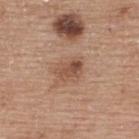follow-up: no biopsy performed (imaged during a skin exam) | body site: the upper back | imaging modality: ~15 mm crop, total-body skin-cancer survey | patient: female, aged around 60 | lighting: white-light | TBP lesion metrics: an average lesion color of about L≈51 a*≈20 b*≈29 (CIELAB) and about 10 CIELAB-L* units darker than the surrounding skin; a border-irregularity rating of about 2/10, a within-lesion color-variation index near 5/10, and a peripheral color-asymmetry measure near 2; a nevus-likeness score of about 50/100 and a lesion-detection confidence of about 100/100.